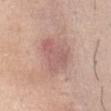| field | value |
|---|---|
| follow-up | total-body-photography surveillance lesion; no biopsy |
| site | the abdomen |
| tile lighting | white-light |
| diameter | ~4.5 mm (longest diameter) |
| image | 15 mm crop, total-body photography |
| patient | female, aged 43–47 |
| TBP lesion metrics | a lesion–skin lightness drop of about 8 and a normalized lesion–skin contrast near 5.5; a color-variation rating of about 3.5/10 and peripheral color asymmetry of about 1.5; a nevus-likeness score of about 10/100 and a detector confidence of about 100 out of 100 that the crop contains a lesion |